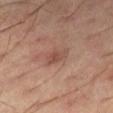Assessment:
Imaged during a routine full-body skin examination; the lesion was not biopsied and no histopathology is available.
Clinical summary:
The lesion is located on the right thigh. A close-up tile cropped from a whole-body skin photograph, about 15 mm across. Automated image analysis of the tile measured an area of roughly 4 mm², a shape eccentricity near 0.8, and a symmetry-axis asymmetry near 0.25. The analysis additionally found a mean CIELAB color near L≈45 a*≈20 b*≈25, roughly 7 lightness units darker than nearby skin, and a normalized border contrast of about 6. The software also gave border irregularity of about 2.5 on a 0–10 scale, internal color variation of about 2 on a 0–10 scale, and radial color variation of about 0.5. The analysis additionally found a lesion-detection confidence of about 100/100. A male subject roughly 60 years of age. Approximately 3 mm at its widest. Captured under cross-polarized illumination.